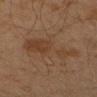The lesion was tiled from a total-body skin photograph and was not biopsied.
The lesion is on the left forearm.
A male patient aged around 45.
A 15 mm close-up extracted from a 3D total-body photography capture.